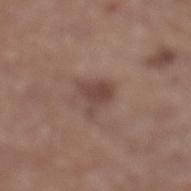notes = catalogued during a skin exam; not biopsied | patient = male, in their mid- to late 60s | TBP lesion metrics = a lesion area of about 7 mm², a shape eccentricity near 0.45, and a symmetry-axis asymmetry near 0.45; peripheral color asymmetry of about 1 | illumination = white-light | body site = the left lower leg | imaging modality = ~15 mm tile from a whole-body skin photo.